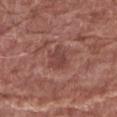{
  "biopsy_status": "not biopsied; imaged during a skin examination",
  "image": {
    "source": "total-body photography crop",
    "field_of_view_mm": 15
  },
  "patient": {
    "sex": "male",
    "age_approx": 80
  },
  "lighting": "white-light",
  "site": "right forearm",
  "lesion_size": {
    "long_diameter_mm_approx": 3.0
  }
}